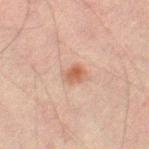Q: What are the patient's age and sex?
A: male, in their 30s
Q: What is the anatomic site?
A: the right thigh
Q: What kind of image is this?
A: ~15 mm tile from a whole-body skin photo
Q: How large is the lesion?
A: ≈3 mm
Q: Illumination type?
A: cross-polarized